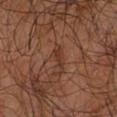Acquisition and patient details:
A male subject, about 65 years old. On the arm. The lesion-visualizer software estimated a lesion area of about 3 mm², an eccentricity of roughly 0.95, and a shape-asymmetry score of about 0.55 (0 = symmetric). And it measured border irregularity of about 6.5 on a 0–10 scale, internal color variation of about 0.5 on a 0–10 scale, and radial color variation of about 0. This image is a 15 mm lesion crop taken from a total-body photograph. Measured at roughly 3 mm in maximum diameter.